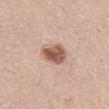Imaged during a routine full-body skin examination; the lesion was not biopsied and no histopathology is available. The lesion is on the front of the torso. The recorded lesion diameter is about 3.5 mm. Captured under white-light illumination. The patient is a male roughly 60 years of age. Cropped from a whole-body photographic skin survey; the tile spans about 15 mm.